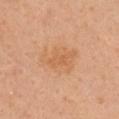The lesion was photographed on a routine skin check and not biopsied; there is no pathology result.
The total-body-photography lesion software estimated a footprint of about 12 mm² and an eccentricity of roughly 0.7. The analysis additionally found a lesion–skin lightness drop of about 6 and a normalized lesion–skin contrast near 5. The software also gave an automated nevus-likeness rating near 5 out of 100 and a lesion-detection confidence of about 100/100.
Measured at roughly 4.5 mm in maximum diameter.
This is a white-light tile.
On the front of the torso.
A female subject roughly 45 years of age.
Cropped from a total-body skin-imaging series; the visible field is about 15 mm.